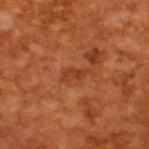Captured during whole-body skin photography for melanoma surveillance; the lesion was not biopsied.
A lesion tile, about 15 mm wide, cut from a 3D total-body photograph.
The tile uses cross-polarized illumination.
The patient is a male in their mid- to late 60s.
Automated tile analysis of the lesion measured a lesion color around L≈40 a*≈29 b*≈38 in CIELAB, roughly 7 lightness units darker than nearby skin, and a normalized lesion–skin contrast near 5.5.